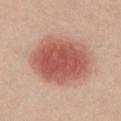notes = catalogued during a skin exam; not biopsied | subject = female, aged 48–52 | location = the chest | tile lighting = white-light | diameter = ≈7.5 mm | image = ~15 mm crop, total-body skin-cancer survey.